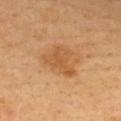The lesion was tiled from a total-body skin photograph and was not biopsied. A lesion tile, about 15 mm wide, cut from a 3D total-body photograph. The patient is a female in their mid- to late 40s. From the chest. Approximately 4.5 mm at its widest.